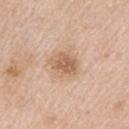This lesion was catalogued during total-body skin photography and was not selected for biopsy. About 3.5 mm across. Imaged with white-light lighting. A 15 mm crop from a total-body photograph taken for skin-cancer surveillance. Located on the left upper arm. A male patient aged around 60.